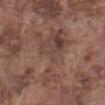Assessment: Recorded during total-body skin imaging; not selected for excision or biopsy. Image and clinical context: The lesion is on the abdomen. A male patient, aged around 75. Cropped from a total-body skin-imaging series; the visible field is about 15 mm. Imaged with white-light lighting. Longest diameter approximately 8 mm.